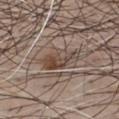biopsy status: catalogued during a skin exam; not biopsied
illumination: white-light illumination
anatomic site: the chest
patient: male, aged around 35
acquisition: 15 mm crop, total-body photography